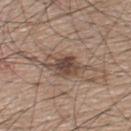Q: Is there a histopathology result?
A: no biopsy performed (imaged during a skin exam)
Q: Where on the body is the lesion?
A: the upper back
Q: Patient demographics?
A: male, aged around 75
Q: How was this image acquired?
A: 15 mm crop, total-body photography